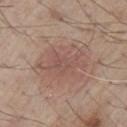biopsy status: imaged on a skin check; not biopsied | tile lighting: white-light illumination | site: the chest | acquisition: ~15 mm tile from a whole-body skin photo | subject: male, about 80 years old | automated lesion analysis: a lesion area of about 21 mm² and an outline eccentricity of about 0.75 (0 = round, 1 = elongated); a mean CIELAB color near L≈53 a*≈19 b*≈24 and a normalized border contrast of about 5 | size: about 6.5 mm.